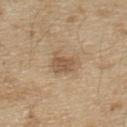• lesion diameter: ≈3.5 mm
• illumination: white-light illumination
• imaging modality: 15 mm crop, total-body photography
• subject: male, aged 68–72
• location: the chest
• image-analysis metrics: an area of roughly 6.5 mm², an outline eccentricity of about 0.6 (0 = round, 1 = elongated), and two-axis asymmetry of about 0.4; a mean CIELAB color near L≈55 a*≈16 b*≈32, roughly 9 lightness units darker than nearby skin, and a lesion-to-skin contrast of about 6.5 (normalized; higher = more distinct); a border-irregularity index near 4/10